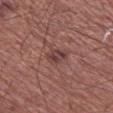From the leg.
A 15 mm close-up extracted from a 3D total-body photography capture.
A male patient aged approximately 65.
Longest diameter approximately 2.5 mm.
Captured under white-light illumination.
The total-body-photography lesion software estimated a lesion area of about 4 mm², an eccentricity of roughly 0.65, and a symmetry-axis asymmetry near 0.25. The analysis additionally found a border-irregularity rating of about 2.5/10 and a peripheral color-asymmetry measure near 1.5.